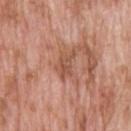Captured during whole-body skin photography for melanoma surveillance; the lesion was not biopsied. A male subject in their 60s. Imaged with white-light lighting. The lesion's longest dimension is about 3.5 mm. On the upper back. Automated image analysis of the tile measured an automated nevus-likeness rating near 0 out of 100 and lesion-presence confidence of about 100/100. Cropped from a total-body skin-imaging series; the visible field is about 15 mm.